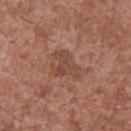Impression:
Recorded during total-body skin imaging; not selected for excision or biopsy.
Image and clinical context:
A male patient aged 43–47. From the upper back. Imaged with white-light lighting. Measured at roughly 4 mm in maximum diameter. An algorithmic analysis of the crop reported a footprint of about 8 mm², an eccentricity of roughly 0.65, and two-axis asymmetry of about 0.55. It also reported a nevus-likeness score of about 0/100. A close-up tile cropped from a whole-body skin photograph, about 15 mm across.